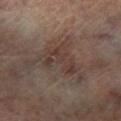Assessment: Part of a total-body skin-imaging series; this lesion was reviewed on a skin check and was not flagged for biopsy. Image and clinical context: Located on the left lower leg. A lesion tile, about 15 mm wide, cut from a 3D total-body photograph. Captured under cross-polarized illumination. The lesion-visualizer software estimated roughly 6 lightness units darker than nearby skin and a lesion-to-skin contrast of about 6 (normalized; higher = more distinct). It also reported an automated nevus-likeness rating near 5 out of 100. A male subject, roughly 65 years of age.